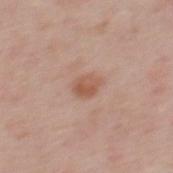Clinical impression:
The lesion was photographed on a routine skin check and not biopsied; there is no pathology result.
Image and clinical context:
The tile uses white-light illumination. Measured at roughly 2.5 mm in maximum diameter. The lesion-visualizer software estimated a footprint of about 4.5 mm², an outline eccentricity of about 0.6 (0 = round, 1 = elongated), and two-axis asymmetry of about 0.2. The software also gave a lesion color around L≈55 a*≈22 b*≈29 in CIELAB, roughly 9 lightness units darker than nearby skin, and a normalized border contrast of about 7. And it measured a border-irregularity rating of about 2/10, a within-lesion color-variation index near 3.5/10, and radial color variation of about 1. It also reported an automated nevus-likeness rating near 45 out of 100 and a lesion-detection confidence of about 100/100. A region of skin cropped from a whole-body photographic capture, roughly 15 mm wide. On the mid back. A male patient approximately 40 years of age.